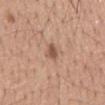<record>
<image>
  <source>total-body photography crop</source>
  <field_of_view_mm>15</field_of_view_mm>
</image>
<patient>
  <sex>male</sex>
  <age_approx>55</age_approx>
</patient>
<lesion_size>
  <long_diameter_mm_approx>3.0</long_diameter_mm_approx>
</lesion_size>
<lighting>white-light</lighting>
<site>mid back</site>
</record>